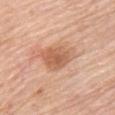Assessment:
The lesion was photographed on a routine skin check and not biopsied; there is no pathology result.
Image and clinical context:
Measured at roughly 4.5 mm in maximum diameter. An algorithmic analysis of the crop reported a lesion color around L≈60 a*≈23 b*≈34 in CIELAB and a lesion-to-skin contrast of about 7 (normalized; higher = more distinct). And it measured a classifier nevus-likeness of about 75/100 and lesion-presence confidence of about 100/100. On the upper back. Cropped from a whole-body photographic skin survey; the tile spans about 15 mm. A female patient approximately 65 years of age. This is a white-light tile.